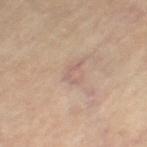Assessment:
The lesion was tiled from a total-body skin photograph and was not biopsied.
Context:
A 15 mm close-up extracted from a 3D total-body photography capture. Captured under cross-polarized illumination. Longest diameter approximately 2.5 mm. Located on the left thigh. The subject is a female roughly 65 years of age.